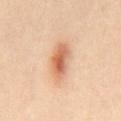Recorded during total-body skin imaging; not selected for excision or biopsy. This is a cross-polarized tile. The lesion is located on the mid back. A 15 mm close-up tile from a total-body photography series done for melanoma screening. The subject is a male aged 33 to 37. About 4 mm across. Automated tile analysis of the lesion measured an area of roughly 9.5 mm², an outline eccentricity of about 0.8 (0 = round, 1 = elongated), and a symmetry-axis asymmetry near 0.2. And it measured about 12 CIELAB-L* units darker than the surrounding skin and a normalized lesion–skin contrast near 8. The software also gave a border-irregularity rating of about 2/10 and internal color variation of about 7.5 on a 0–10 scale.